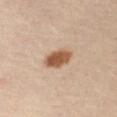A 15 mm crop from a total-body photograph taken for skin-cancer surveillance.
The lesion is located on the right upper arm.
The recorded lesion diameter is about 3.5 mm.
Automated image analysis of the tile measured an average lesion color of about L≈54 a*≈21 b*≈32 (CIELAB), a lesion–skin lightness drop of about 15, and a lesion-to-skin contrast of about 10.5 (normalized; higher = more distinct). It also reported an automated nevus-likeness rating near 100 out of 100 and a lesion-detection confidence of about 100/100.
The subject is approximately 55 years of age.
Imaged with cross-polarized lighting.